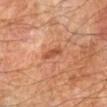biopsy status — catalogued during a skin exam; not biopsied
body site — the arm
acquisition — ~15 mm crop, total-body skin-cancer survey
automated metrics — an average lesion color of about L≈51 a*≈28 b*≈36 (CIELAB); border irregularity of about 3.5 on a 0–10 scale and a peripheral color-asymmetry measure near 0
patient — male, roughly 70 years of age
diameter — ~3 mm (longest diameter)